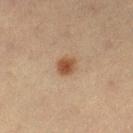Q: Was a biopsy performed?
A: catalogued during a skin exam; not biopsied
Q: Automated lesion metrics?
A: a footprint of about 4.5 mm² and a symmetry-axis asymmetry near 0.2; a border-irregularity rating of about 1.5/10
Q: Patient demographics?
A: female, aged around 20
Q: How was the tile lit?
A: cross-polarized
Q: Lesion size?
A: ~2.5 mm (longest diameter)
Q: Lesion location?
A: the left lower leg
Q: What kind of image is this?
A: ~15 mm crop, total-body skin-cancer survey